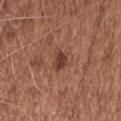The lesion was photographed on a routine skin check and not biopsied; there is no pathology result. The tile uses white-light illumination. The subject is a male roughly 50 years of age. A close-up tile cropped from a whole-body skin photograph, about 15 mm across. The recorded lesion diameter is about 3 mm. An algorithmic analysis of the crop reported a border-irregularity rating of about 3.5/10, a within-lesion color-variation index near 2/10, and radial color variation of about 0.5. From the head or neck.